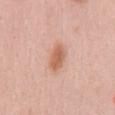workup=total-body-photography surveillance lesion; no biopsy.